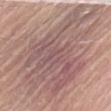workup: catalogued during a skin exam; not biopsied
site: the right thigh
TBP lesion metrics: a lesion area of about 70 mm², an eccentricity of roughly 0.75, and a shape-asymmetry score of about 0.4 (0 = symmetric); a lesion color around L≈56 a*≈18 b*≈20 in CIELAB, roughly 9 lightness units darker than nearby skin, and a lesion-to-skin contrast of about 7 (normalized; higher = more distinct); border irregularity of about 8 on a 0–10 scale, internal color variation of about 5.5 on a 0–10 scale, and peripheral color asymmetry of about 2
tile lighting: white-light illumination
image source: ~15 mm crop, total-body skin-cancer survey
patient: male, aged 78–82